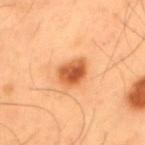• workup: no biopsy performed (imaged during a skin exam)
• image-analysis metrics: an area of roughly 7 mm² and an outline eccentricity of about 0.7 (0 = round, 1 = elongated); peripheral color asymmetry of about 1.5
• image: 15 mm crop, total-body photography
• patient: male, aged 53–57
• tile lighting: cross-polarized illumination
• lesion diameter: ~3.5 mm (longest diameter)
• site: the upper back